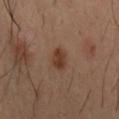Q: Was this lesion biopsied?
A: imaged on a skin check; not biopsied
Q: Lesion size?
A: ≈3 mm
Q: Lesion location?
A: the back
Q: Patient demographics?
A: male, approximately 40 years of age
Q: What kind of image is this?
A: ~15 mm crop, total-body skin-cancer survey
Q: What did automated image analysis measure?
A: a footprint of about 5 mm², an eccentricity of roughly 0.85, and two-axis asymmetry of about 0.3; lesion-presence confidence of about 100/100